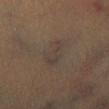Assessment: Recorded during total-body skin imaging; not selected for excision or biopsy. Background: Longest diameter approximately 4 mm. A male patient, in their mid-40s. A roughly 15 mm field-of-view crop from a total-body skin photograph. The lesion is located on the right lower leg. An algorithmic analysis of the crop reported an area of roughly 6.5 mm², an eccentricity of roughly 0.85, and a symmetry-axis asymmetry near 0.45. The software also gave an automated nevus-likeness rating near 0 out of 100 and a lesion-detection confidence of about 50/100.